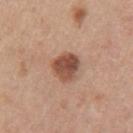Assessment:
No biopsy was performed on this lesion — it was imaged during a full skin examination and was not determined to be concerning.
Background:
The tile uses white-light illumination. A female patient, aged around 45. A 15 mm close-up tile from a total-body photography series done for melanoma screening. An algorithmic analysis of the crop reported a footprint of about 8 mm², a shape eccentricity near 0.4, and a shape-asymmetry score of about 0.2 (0 = symmetric). It also reported a border-irregularity index near 2/10 and radial color variation of about 1.5. The lesion's longest dimension is about 3 mm. Located on the left upper arm.